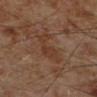{"biopsy_status": "not biopsied; imaged during a skin examination", "automated_metrics": {"area_mm2_approx": 9.0, "eccentricity": 0.9, "shape_asymmetry": 0.5, "cielab_L": 35, "cielab_a": 18, "cielab_b": 28, "vs_skin_darker_L": 6.0, "vs_skin_contrast_norm": 6.0, "color_variation_0_10": 1.5, "peripheral_color_asymmetry": 0.5, "nevus_likeness_0_100": 0, "lesion_detection_confidence_0_100": 100}, "lesion_size": {"long_diameter_mm_approx": 5.5}, "patient": {"sex": "male", "age_approx": 70}, "lighting": "cross-polarized", "site": "left lower leg", "image": {"source": "total-body photography crop", "field_of_view_mm": 15}}